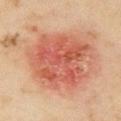The lesion was tiled from a total-body skin photograph and was not biopsied.
Longest diameter approximately 8 mm.
A female subject aged around 35.
The lesion-visualizer software estimated a lesion color around L≈56 a*≈30 b*≈32 in CIELAB, about 11 CIELAB-L* units darker than the surrounding skin, and a lesion-to-skin contrast of about 7 (normalized; higher = more distinct). It also reported a within-lesion color-variation index near 6.5/10.
Located on the right upper arm.
Cropped from a whole-body photographic skin survey; the tile spans about 15 mm.
This is a cross-polarized tile.